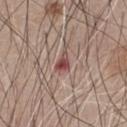Imaged during a routine full-body skin examination; the lesion was not biopsied and no histopathology is available.
Automated tile analysis of the lesion measured border irregularity of about 4.5 on a 0–10 scale.
A male subject roughly 65 years of age.
Measured at roughly 2.5 mm in maximum diameter.
A close-up tile cropped from a whole-body skin photograph, about 15 mm across.
Located on the front of the torso.